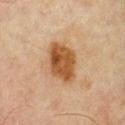| field | value |
|---|---|
| lighting | cross-polarized |
| subject | male, roughly 70 years of age |
| anatomic site | the chest |
| imaging modality | ~15 mm crop, total-body skin-cancer survey |
| size | about 5 mm |